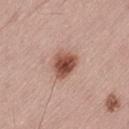Q: Was this lesion biopsied?
A: imaged on a skin check; not biopsied
Q: What is the imaging modality?
A: ~15 mm tile from a whole-body skin photo
Q: Automated lesion metrics?
A: an automated nevus-likeness rating near 100 out of 100 and a lesion-detection confidence of about 100/100
Q: Lesion size?
A: ≈3.5 mm
Q: Who is the patient?
A: male, approximately 55 years of age
Q: Lesion location?
A: the left thigh
Q: What lighting was used for the tile?
A: white-light illumination